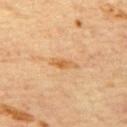  biopsy_status: not biopsied; imaged during a skin examination
  automated_metrics:
    cielab_L: 56
    cielab_a: 20
    cielab_b: 39
    vs_skin_darker_L: 8.0
    vs_skin_contrast_norm: 6.5
    nevus_likeness_0_100: 0
  patient:
    sex: male
    age_approx: 65
  image:
    source: total-body photography crop
    field_of_view_mm: 15
  lesion_size:
    long_diameter_mm_approx: 3.0
  site: back